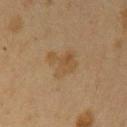notes: catalogued during a skin exam; not biopsied | TBP lesion metrics: a footprint of about 9 mm², a shape eccentricity near 0.7, and a shape-asymmetry score of about 0.35 (0 = symmetric); a lesion color around L≈40 a*≈13 b*≈29 in CIELAB and a normalized border contrast of about 5.5; a border-irregularity rating of about 4.5/10, internal color variation of about 2.5 on a 0–10 scale, and radial color variation of about 1; a lesion-detection confidence of about 100/100 | subject: female, aged 38 to 42 | size: about 4 mm | illumination: cross-polarized illumination | anatomic site: the left upper arm | image: total-body-photography crop, ~15 mm field of view.